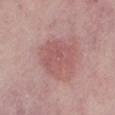| field | value |
|---|---|
| biopsy status | no biopsy performed (imaged during a skin exam) |
| lighting | white-light |
| anatomic site | the left lower leg |
| image-analysis metrics | internal color variation of about 3 on a 0–10 scale and peripheral color asymmetry of about 1; a lesion-detection confidence of about 100/100 |
| lesion size | ≈5 mm |
| imaging modality | ~15 mm crop, total-body skin-cancer survey |
| patient | female, aged 63–67 |